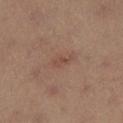imaging modality — ~15 mm tile from a whole-body skin photo | illumination — cross-polarized | subject — female, aged around 35 | body site — the leg | size — ≈2.5 mm | image-analysis metrics — a lesion color around L≈46 a*≈20 b*≈26 in CIELAB and a lesion–skin lightness drop of about 6.